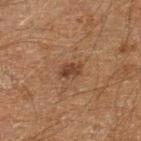Assessment: Imaged during a routine full-body skin examination; the lesion was not biopsied and no histopathology is available.